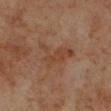biopsy status: imaged on a skin check; not biopsied